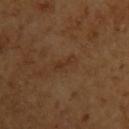Findings:
– workup — imaged on a skin check; not biopsied
– automated metrics — a lesion color around L≈33 a*≈19 b*≈30 in CIELAB and roughly 5 lightness units darker than nearby skin; a border-irregularity index near 4.5/10, internal color variation of about 0 on a 0–10 scale, and a peripheral color-asymmetry measure near 0
– site — the left upper arm
– patient — female, aged 53 to 57
– illumination — cross-polarized illumination
– diameter — ~3 mm (longest diameter)
– acquisition — total-body-photography crop, ~15 mm field of view